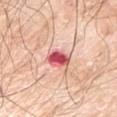Impression: No biopsy was performed on this lesion — it was imaged during a full skin examination and was not determined to be concerning. Clinical summary: A male patient aged around 70. The tile uses white-light illumination. About 3 mm across. A close-up tile cropped from a whole-body skin photograph, about 15 mm across. On the arm.